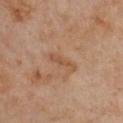Notes:
- follow-up · total-body-photography surveillance lesion; no biopsy
- acquisition · total-body-photography crop, ~15 mm field of view
- patient · male, approximately 55 years of age
- illumination · cross-polarized
- location · the chest
- lesion diameter · ≈3.5 mm
- automated metrics · a mean CIELAB color near L≈52 a*≈21 b*≈33 and roughly 7 lightness units darker than nearby skin; border irregularity of about 5.5 on a 0–10 scale, internal color variation of about 0 on a 0–10 scale, and peripheral color asymmetry of about 0